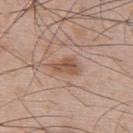Captured during whole-body skin photography for melanoma surveillance; the lesion was not biopsied. The lesion-visualizer software estimated a lesion area of about 5 mm², a shape eccentricity near 0.8, and a shape-asymmetry score of about 0.35 (0 = symmetric). The analysis additionally found a border-irregularity index near 3.5/10 and a color-variation rating of about 3.5/10. Captured under white-light illumination. Approximately 3.5 mm at its widest. A male patient aged approximately 55. From the upper back. A region of skin cropped from a whole-body photographic capture, roughly 15 mm wide.